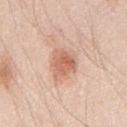  biopsy_status: not biopsied; imaged during a skin examination
  lighting: white-light
  site: chest
  lesion_size:
    long_diameter_mm_approx: 3.5
  image:
    source: total-body photography crop
    field_of_view_mm: 15
  patient:
    sex: male
    age_approx: 45